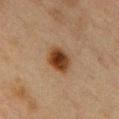| key | value |
|---|---|
| workup | total-body-photography surveillance lesion; no biopsy |
| imaging modality | ~15 mm tile from a whole-body skin photo |
| patient | female, about 40 years old |
| illumination | cross-polarized |
| lesion diameter | about 3.5 mm |
| automated metrics | an area of roughly 8.5 mm², an eccentricity of roughly 0.65, and a symmetry-axis asymmetry near 0.1; about 13 CIELAB-L* units darker than the surrounding skin and a normalized border contrast of about 12; a border-irregularity index near 1/10 and peripheral color asymmetry of about 1.5; a classifier nevus-likeness of about 100/100 and a lesion-detection confidence of about 100/100 |
| body site | the chest |